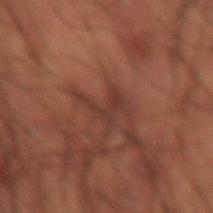workup: total-body-photography surveillance lesion; no biopsy | anatomic site: the right thigh | patient: male, aged 48–52 | imaging modality: 15 mm crop, total-body photography | automated lesion analysis: a shape eccentricity near 0.75 and a symmetry-axis asymmetry near 0.75; a mean CIELAB color near L≈27 a*≈18 b*≈20 and a lesion–skin lightness drop of about 5; a border-irregularity index near 9.5/10, a color-variation rating of about 1.5/10, and radial color variation of about 0.5 | size: ~4 mm (longest diameter) | tile lighting: cross-polarized.